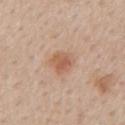Case summary:
– workup: total-body-photography surveillance lesion; no biopsy
– lesion size: ≈3 mm
– acquisition: 15 mm crop, total-body photography
– patient: male, aged 58–62
– site: the mid back
– TBP lesion metrics: an average lesion color of about L≈58 a*≈21 b*≈31 (CIELAB), a lesion–skin lightness drop of about 9, and a lesion-to-skin contrast of about 6.5 (normalized; higher = more distinct); an automated nevus-likeness rating near 80 out of 100 and lesion-presence confidence of about 100/100